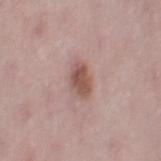biopsy status: no biopsy performed (imaged during a skin exam); patient: female, approximately 50 years of age; lesion diameter: ~3.5 mm (longest diameter); image source: total-body-photography crop, ~15 mm field of view; anatomic site: the right thigh; tile lighting: white-light illumination.